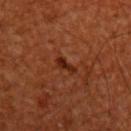follow-up: total-body-photography surveillance lesion; no biopsy
site: the upper back
patient: male, approximately 60 years of age
automated lesion analysis: a footprint of about 3 mm² and an eccentricity of roughly 0.9; a lesion color around L≈25 a*≈25 b*≈29 in CIELAB and a lesion–skin lightness drop of about 8; a border-irregularity rating of about 5/10, internal color variation of about 1.5 on a 0–10 scale, and a peripheral color-asymmetry measure near 0.5; a nevus-likeness score of about 70/100 and lesion-presence confidence of about 100/100
size: about 3 mm
acquisition: 15 mm crop, total-body photography
illumination: cross-polarized